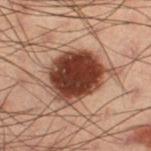body site = the right thigh; patient = male, in their mid- to late 50s; tile lighting = cross-polarized; imaging modality = ~15 mm crop, total-body skin-cancer survey; size = ~5.5 mm (longest diameter).